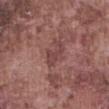| feature | finding |
|---|---|
| follow-up | catalogued during a skin exam; not biopsied |
| body site | the abdomen |
| tile lighting | white-light |
| imaging modality | 15 mm crop, total-body photography |
| subject | male, aged around 75 |
| diameter | ≈3.5 mm |